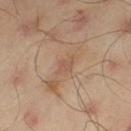<tbp_lesion>
<biopsy_status>not biopsied; imaged during a skin examination</biopsy_status>
<site>left thigh</site>
<lighting>cross-polarized</lighting>
<patient>
  <sex>male</sex>
  <age_approx>45</age_approx>
</patient>
<lesion_size>
  <long_diameter_mm_approx>3.0</long_diameter_mm_approx>
</lesion_size>
<image>
  <source>total-body photography crop</source>
  <field_of_view_mm>15</field_of_view_mm>
</image>
</tbp_lesion>